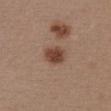Impression: The lesion was photographed on a routine skin check and not biopsied; there is no pathology result. Image and clinical context: A 15 mm crop from a total-body photograph taken for skin-cancer surveillance. The patient is a female aged 28–32. The lesion is on the chest. Longest diameter approximately 3 mm. The lesion-visualizer software estimated an area of roughly 6 mm², an outline eccentricity of about 0.45 (0 = round, 1 = elongated), and a symmetry-axis asymmetry near 0.2. It also reported a normalized lesion–skin contrast near 9.5.